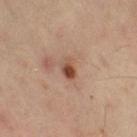Q: Is there a histopathology result?
A: imaged on a skin check; not biopsied
Q: Patient demographics?
A: female, aged around 40
Q: What did automated image analysis measure?
A: an area of roughly 4 mm², a shape eccentricity near 0.8, and a symmetry-axis asymmetry near 0.2; an average lesion color of about L≈49 a*≈21 b*≈30 (CIELAB), roughly 12 lightness units darker than nearby skin, and a normalized lesion–skin contrast near 9; a color-variation rating of about 8.5/10 and peripheral color asymmetry of about 3
Q: Lesion location?
A: the right thigh
Q: What is the imaging modality?
A: ~15 mm tile from a whole-body skin photo